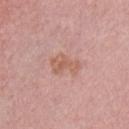Captured during whole-body skin photography for melanoma surveillance; the lesion was not biopsied. The recorded lesion diameter is about 3.5 mm. On the front of the torso. A male subject in their 50s. Cropped from a total-body skin-imaging series; the visible field is about 15 mm. The tile uses white-light illumination.